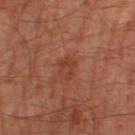Q: What are the patient's age and sex?
A: male, roughly 65 years of age
Q: What is the lesion's diameter?
A: about 2.5 mm
Q: Lesion location?
A: the right thigh
Q: How was this image acquired?
A: ~15 mm tile from a whole-body skin photo